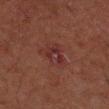Clinical impression:
This lesion was catalogued during total-body skin photography and was not selected for biopsy.
Background:
The tile uses cross-polarized illumination. Measured at roughly 3.5 mm in maximum diameter. A close-up tile cropped from a whole-body skin photograph, about 15 mm across. A male patient approximately 60 years of age. On the left upper arm.